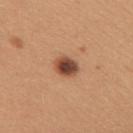<record>
  <biopsy_status>not biopsied; imaged during a skin examination</biopsy_status>
  <image>
    <source>total-body photography crop</source>
    <field_of_view_mm>15</field_of_view_mm>
  </image>
  <lighting>white-light</lighting>
  <patient>
    <sex>female</sex>
    <age_approx>30</age_approx>
  </patient>
  <site>upper back</site>
  <lesion_size>
    <long_diameter_mm_approx>3.0</long_diameter_mm_approx>
  </lesion_size>
</record>